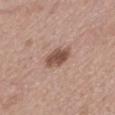Part of a total-body skin-imaging series; this lesion was reviewed on a skin check and was not flagged for biopsy. A male subject in their mid-50s. A lesion tile, about 15 mm wide, cut from a 3D total-body photograph.